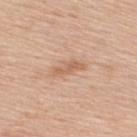Recorded during total-body skin imaging; not selected for excision or biopsy.
This is a white-light tile.
A region of skin cropped from a whole-body photographic capture, roughly 15 mm wide.
The lesion's longest dimension is about 3.5 mm.
The patient is a male roughly 50 years of age.
Located on the upper back.
An algorithmic analysis of the crop reported a lesion area of about 5 mm² and a symmetry-axis asymmetry near 0.3. The software also gave an average lesion color of about L≈62 a*≈20 b*≈32 (CIELAB), roughly 9 lightness units darker than nearby skin, and a lesion-to-skin contrast of about 6 (normalized; higher = more distinct). And it measured a color-variation rating of about 2/10 and radial color variation of about 0.5.